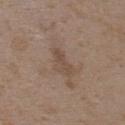A 15 mm close-up tile from a total-body photography series done for melanoma screening. The total-body-photography lesion software estimated a footprint of about 4 mm², an eccentricity of roughly 0.9, and two-axis asymmetry of about 0.3. A female subject in their mid- to late 30s. The lesion is on the upper back. Captured under white-light illumination.